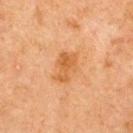Clinical impression:
This lesion was catalogued during total-body skin photography and was not selected for biopsy.
Context:
The tile uses cross-polarized illumination. Located on the upper back. Measured at roughly 3.5 mm in maximum diameter. A 15 mm close-up extracted from a 3D total-body photography capture. A female patient in their 60s.